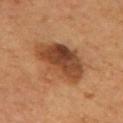biopsy status — catalogued during a skin exam; not biopsied
site — the back
image-analysis metrics — a lesion area of about 23 mm², an eccentricity of roughly 0.7, and two-axis asymmetry of about 0.25; roughly 12 lightness units darker than nearby skin and a lesion-to-skin contrast of about 9 (normalized; higher = more distinct); a color-variation rating of about 7/10 and radial color variation of about 2; a classifier nevus-likeness of about 70/100 and a lesion-detection confidence of about 100/100
tile lighting — cross-polarized illumination
image — ~15 mm tile from a whole-body skin photo
patient — male, aged around 55
diameter — about 6.5 mm